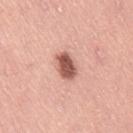image:
  source: total-body photography crop
  field_of_view_mm: 15
lesion_size:
  long_diameter_mm_approx: 3.5
patient:
  sex: female
  age_approx: 40
automated_metrics:
  eccentricity: 0.8
  shape_asymmetry: 0.2
  border_irregularity_0_10: 1.5
  color_variation_0_10: 3.5
  peripheral_color_asymmetry: 1.0
  nevus_likeness_0_100: 100
  lesion_detection_confidence_0_100: 100
site: right thigh